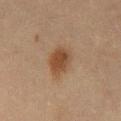| feature | finding |
|---|---|
| image | ~15 mm tile from a whole-body skin photo |
| body site | the front of the torso |
| patient | male, in their mid-40s |